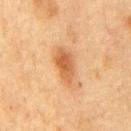biopsy status: catalogued during a skin exam; not biopsied
site: the chest
patient: male, roughly 75 years of age
size: ~4.5 mm (longest diameter)
imaging modality: total-body-photography crop, ~15 mm field of view